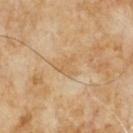Impression:
No biopsy was performed on this lesion — it was imaged during a full skin examination and was not determined to be concerning.
Background:
A roughly 15 mm field-of-view crop from a total-body skin photograph. A male subject roughly 65 years of age.